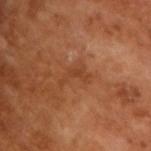Assessment: Part of a total-body skin-imaging series; this lesion was reviewed on a skin check and was not flagged for biopsy. Context: Cropped from a total-body skin-imaging series; the visible field is about 15 mm. Automated tile analysis of the lesion measured border irregularity of about 8 on a 0–10 scale, internal color variation of about 0.5 on a 0–10 scale, and radial color variation of about 0. And it measured a lesion-detection confidence of about 100/100. A male subject aged 63–67. The lesion's longest dimension is about 3.5 mm. The tile uses cross-polarized illumination.